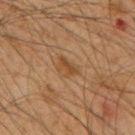This lesion was catalogued during total-body skin photography and was not selected for biopsy.
The subject is a male aged approximately 65.
About 3 mm across.
The lesion is on the upper back.
Cropped from a whole-body photographic skin survey; the tile spans about 15 mm.
The total-body-photography lesion software estimated a lesion color around L≈38 a*≈16 b*≈30 in CIELAB, roughly 6 lightness units darker than nearby skin, and a normalized border contrast of about 6. It also reported a color-variation rating of about 3/10 and peripheral color asymmetry of about 1.